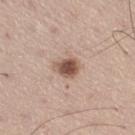| key | value |
|---|---|
| workup | no biopsy performed (imaged during a skin exam) |
| subject | male, aged 58 to 62 |
| lesion size | ~3 mm (longest diameter) |
| site | the leg |
| illumination | white-light |
| image | ~15 mm crop, total-body skin-cancer survey |